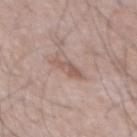Recorded during total-body skin imaging; not selected for excision or biopsy. The lesion is located on the chest. Cropped from a whole-body photographic skin survey; the tile spans about 15 mm. The patient is a male aged around 70.